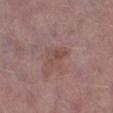Background:
The tile uses white-light illumination. About 3 mm across. From the left lower leg. A 15 mm close-up tile from a total-body photography series done for melanoma screening. The lesion-visualizer software estimated a lesion area of about 4.5 mm², an eccentricity of roughly 0.8, and two-axis asymmetry of about 0.3. It also reported an average lesion color of about L≈47 a*≈20 b*≈22 (CIELAB) and roughly 6 lightness units darker than nearby skin. The software also gave an automated nevus-likeness rating near 0 out of 100 and lesion-presence confidence of about 100/100. The patient is a male aged around 55.